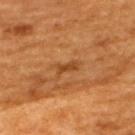Recorded during total-body skin imaging; not selected for excision or biopsy. A roughly 15 mm field-of-view crop from a total-body skin photograph. On the upper back. The patient is a male aged around 60.